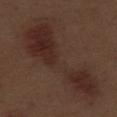patient:
  sex: male
  age_approx: 70
automated_metrics:
  area_mm2_approx: 45.0
  eccentricity: 0.95
  shape_asymmetry: 0.4
  cielab_L: 28
  cielab_a: 17
  cielab_b: 21
  vs_skin_contrast_norm: 7.0
site: leg
image:
  source: total-body photography crop
  field_of_view_mm: 15
lesion_size:
  long_diameter_mm_approx: 13.0
lighting: white-light